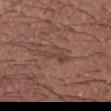Part of a total-body skin-imaging series; this lesion was reviewed on a skin check and was not flagged for biopsy. From the head or neck. A male subject, aged around 25. A 15 mm close-up extracted from a 3D total-body photography capture.